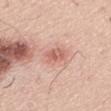Imaged during a routine full-body skin examination; the lesion was not biopsied and no histopathology is available.